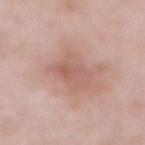follow-up: total-body-photography surveillance lesion; no biopsy
patient: male, in their mid- to late 50s
TBP lesion metrics: a footprint of about 12 mm² and an outline eccentricity of about 0.8 (0 = round, 1 = elongated); border irregularity of about 4 on a 0–10 scale, internal color variation of about 3 on a 0–10 scale, and a peripheral color-asymmetry measure near 1; an automated nevus-likeness rating near 0 out of 100 and a detector confidence of about 100 out of 100 that the crop contains a lesion
image source: total-body-photography crop, ~15 mm field of view
illumination: white-light illumination
lesion size: about 5 mm
body site: the abdomen